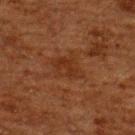No biopsy was performed on this lesion — it was imaged during a full skin examination and was not determined to be concerning.
The lesion is on the upper back.
The total-body-photography lesion software estimated a shape eccentricity near 0.6 and two-axis asymmetry of about 0.25. The software also gave a lesion–skin lightness drop of about 5 and a normalized border contrast of about 5.5. The analysis additionally found a classifier nevus-likeness of about 0/100 and lesion-presence confidence of about 100/100.
A lesion tile, about 15 mm wide, cut from a 3D total-body photograph.
About 3.5 mm across.
Imaged with cross-polarized lighting.
A male patient approximately 60 years of age.